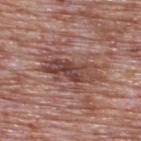Clinical impression: Imaged during a routine full-body skin examination; the lesion was not biopsied and no histopathology is available. Acquisition and patient details: A close-up tile cropped from a whole-body skin photograph, about 15 mm across. Captured under white-light illumination. From the upper back. Automated image analysis of the tile measured a footprint of about 17 mm². The analysis additionally found a border-irregularity rating of about 7/10, a color-variation rating of about 7/10, and radial color variation of about 2.5. A male patient, in their 70s.